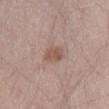Case summary:
• follow-up: total-body-photography surveillance lesion; no biopsy
• acquisition: total-body-photography crop, ~15 mm field of view
• illumination: white-light
• lesion diameter: ~3 mm (longest diameter)
• patient: male, in their 30s